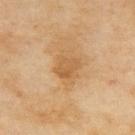workup: imaged on a skin check; not biopsied
site: the upper back
subject: female, aged around 60
image: total-body-photography crop, ~15 mm field of view